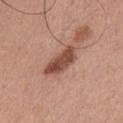Notes:
– notes — total-body-photography surveillance lesion; no biopsy
– site — the chest
– subject — male, about 30 years old
– acquisition — 15 mm crop, total-body photography